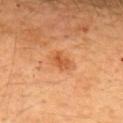Recorded during total-body skin imaging; not selected for excision or biopsy. Longest diameter approximately 2.5 mm. A lesion tile, about 15 mm wide, cut from a 3D total-body photograph. The lesion-visualizer software estimated a mean CIELAB color near L≈55 a*≈29 b*≈43 and a normalized border contrast of about 6.5. It also reported a border-irregularity rating of about 2.5/10, a color-variation rating of about 3/10, and peripheral color asymmetry of about 1. A male patient, aged 28–32. The lesion is located on the upper back.